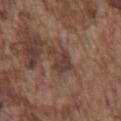No biopsy was performed on this lesion — it was imaged during a full skin examination and was not determined to be concerning.
The lesion-visualizer software estimated a lesion area of about 7 mm², a shape eccentricity near 0.7, and two-axis asymmetry of about 0.45.
This is a white-light tile.
The subject is a male aged 73–77.
A roughly 15 mm field-of-view crop from a total-body skin photograph.
The recorded lesion diameter is about 4 mm.
On the chest.